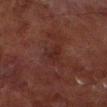| key | value |
|---|---|
| follow-up | catalogued during a skin exam; not biopsied |
| size | ≈2.5 mm |
| body site | the left lower leg |
| imaging modality | ~15 mm tile from a whole-body skin photo |
| subject | male, aged approximately 70 |